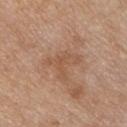The lesion was tiled from a total-body skin photograph and was not biopsied. A male patient, in their mid-50s. The lesion is located on the chest. The recorded lesion diameter is about 4.5 mm. Captured under white-light illumination. Cropped from a whole-body photographic skin survey; the tile spans about 15 mm.